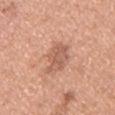| field | value |
|---|---|
| biopsy status | no biopsy performed (imaged during a skin exam) |